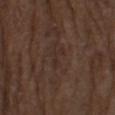No biopsy was performed on this lesion — it was imaged during a full skin examination and was not determined to be concerning. Imaged with white-light lighting. Longest diameter approximately 3 mm. The patient is a male in their mid- to late 70s. From the left thigh. Cropped from a total-body skin-imaging series; the visible field is about 15 mm. Automated image analysis of the tile measured a lesion area of about 2 mm² and a shape-asymmetry score of about 0.5 (0 = symmetric). The software also gave a mean CIELAB color near L≈28 a*≈15 b*≈21, roughly 4 lightness units darker than nearby skin, and a lesion-to-skin contrast of about 5 (normalized; higher = more distinct). The software also gave a border-irregularity rating of about 6.5/10. The software also gave a detector confidence of about 95 out of 100 that the crop contains a lesion.